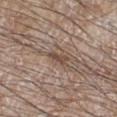follow-up=total-body-photography surveillance lesion; no biopsy | tile lighting=white-light | image=~15 mm crop, total-body skin-cancer survey | anatomic site=the leg | diameter=about 3 mm | patient=male, aged 58–62.